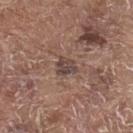Part of a total-body skin-imaging series; this lesion was reviewed on a skin check and was not flagged for biopsy. Located on the upper back. Cropped from a whole-body photographic skin survey; the tile spans about 15 mm. Captured under white-light illumination. A male patient aged approximately 80.